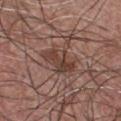  biopsy_status: not biopsied; imaged during a skin examination
  patient:
    sex: male
    age_approx: 50
  automated_metrics:
    area_mm2_approx: 10.0
    eccentricity: 0.85
    shape_asymmetry: 0.25
    nevus_likeness_0_100: 10
    lesion_detection_confidence_0_100: 95
  image:
    source: total-body photography crop
    field_of_view_mm: 15
  site: front of the torso
  lesion_size:
    long_diameter_mm_approx: 5.0